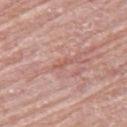subject = female, in their mid- to late 60s | site = the back | imaging modality = ~15 mm crop, total-body skin-cancer survey.